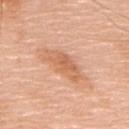Notes:
– workup — total-body-photography surveillance lesion; no biopsy
– body site — the mid back
– patient — male, aged approximately 75
– image — 15 mm crop, total-body photography
– lesion size — about 5.5 mm
– lighting — white-light illumination